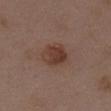Case summary:
– workup: no biopsy performed (imaged during a skin exam)
– site: the chest
– image: 15 mm crop, total-body photography
– automated lesion analysis: an area of roughly 8.5 mm² and a shape-asymmetry score of about 0.15 (0 = symmetric); about 9 CIELAB-L* units darker than the surrounding skin and a lesion-to-skin contrast of about 8 (normalized; higher = more distinct); an automated nevus-likeness rating near 90 out of 100 and a detector confidence of about 100 out of 100 that the crop contains a lesion
– patient: female, aged 33–37
– tile lighting: white-light
– size: ≈3.5 mm